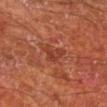Notes:
• follow-up · total-body-photography surveillance lesion; no biopsy
• illumination · cross-polarized illumination
• automated lesion analysis · a shape eccentricity near 0.45 and a symmetry-axis asymmetry near 0.55; a lesion color around L≈39 a*≈29 b*≈32 in CIELAB, a lesion–skin lightness drop of about 7, and a lesion-to-skin contrast of about 6 (normalized; higher = more distinct); a border-irregularity index near 5.5/10 and internal color variation of about 3 on a 0–10 scale
• location · the right lower leg
• diameter · ≈3 mm
• patient · male, aged 63 to 67
• image · total-body-photography crop, ~15 mm field of view